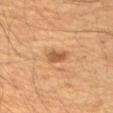Clinical impression:
No biopsy was performed on this lesion — it was imaged during a full skin examination and was not determined to be concerning.
Clinical summary:
A region of skin cropped from a whole-body photographic capture, roughly 15 mm wide. The recorded lesion diameter is about 3 mm. From the right upper arm. Automated image analysis of the tile measured an average lesion color of about L≈51 a*≈21 b*≈36 (CIELAB), about 11 CIELAB-L* units darker than the surrounding skin, and a lesion-to-skin contrast of about 7.5 (normalized; higher = more distinct). It also reported a border-irregularity index near 2.5/10, internal color variation of about 2 on a 0–10 scale, and radial color variation of about 0.5. A male patient approximately 55 years of age.